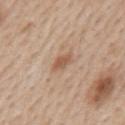{"biopsy_status": "not biopsied; imaged during a skin examination", "lesion_size": {"long_diameter_mm_approx": 3.0}, "image": {"source": "total-body photography crop", "field_of_view_mm": 15}, "site": "mid back", "lighting": "white-light", "patient": {"sex": "male", "age_approx": 60}}